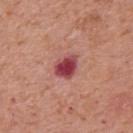  biopsy_status: not biopsied; imaged during a skin examination
  patient:
    sex: male
    age_approx: 70
  lighting: white-light
  image:
    source: total-body photography crop
    field_of_view_mm: 15
  site: upper back
  lesion_size:
    long_diameter_mm_approx: 3.5